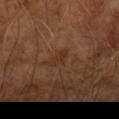Q: Is there a histopathology result?
A: imaged on a skin check; not biopsied
Q: How large is the lesion?
A: ~2.5 mm (longest diameter)
Q: What lighting was used for the tile?
A: cross-polarized
Q: What did automated image analysis measure?
A: a footprint of about 3.5 mm², an outline eccentricity of about 0.8 (0 = round, 1 = elongated), and a symmetry-axis asymmetry near 0.3; an average lesion color of about L≈31 a*≈19 b*≈29 (CIELAB); a classifier nevus-likeness of about 0/100 and a detector confidence of about 100 out of 100 that the crop contains a lesion
Q: How was this image acquired?
A: 15 mm crop, total-body photography
Q: Patient demographics?
A: male, roughly 65 years of age